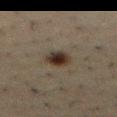follow-up: total-body-photography surveillance lesion; no biopsy
imaging modality: ~15 mm tile from a whole-body skin photo
lesion size: ~3 mm (longest diameter)
subject: male, aged 48–52
lighting: cross-polarized
body site: the abdomen